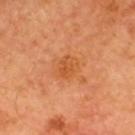| field | value |
|---|---|
| follow-up | imaged on a skin check; not biopsied |
| imaging modality | 15 mm crop, total-body photography |
| site | the upper back |
| subject | male, in their mid- to late 60s |